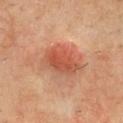Q: Was this lesion biopsied?
A: no biopsy performed (imaged during a skin exam)
Q: What is the lesion's diameter?
A: ~5 mm (longest diameter)
Q: What are the patient's age and sex?
A: male, roughly 70 years of age
Q: What did automated image analysis measure?
A: an automated nevus-likeness rating near 80 out of 100 and a lesion-detection confidence of about 100/100
Q: Lesion location?
A: the chest
Q: What is the imaging modality?
A: total-body-photography crop, ~15 mm field of view